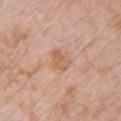Recorded during total-body skin imaging; not selected for excision or biopsy.
The lesion is on the chest.
This image is a 15 mm lesion crop taken from a total-body photograph.
Measured at roughly 2.5 mm in maximum diameter.
Captured under white-light illumination.
A female patient aged approximately 70.